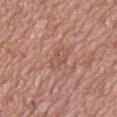Q: Was a biopsy performed?
A: catalogued during a skin exam; not biopsied
Q: What is the anatomic site?
A: the chest
Q: Lesion size?
A: ≈3 mm
Q: Patient demographics?
A: male, in their mid- to late 60s
Q: Automated lesion metrics?
A: a lesion color around L≈53 a*≈22 b*≈28 in CIELAB, a lesion–skin lightness drop of about 6, and a lesion-to-skin contrast of about 4.5 (normalized; higher = more distinct); border irregularity of about 3 on a 0–10 scale, a within-lesion color-variation index near 1.5/10, and radial color variation of about 0.5; an automated nevus-likeness rating near 0 out of 100 and a lesion-detection confidence of about 85/100
Q: What lighting was used for the tile?
A: white-light illumination
Q: How was this image acquired?
A: 15 mm crop, total-body photography